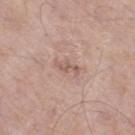| feature | finding |
|---|---|
| workup | total-body-photography surveillance lesion; no biopsy |